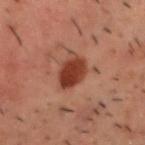<record>
  <lesion_size>
    <long_diameter_mm_approx>3.0</long_diameter_mm_approx>
  </lesion_size>
  <site>head or neck</site>
  <automated_metrics>
    <area_mm2_approx>8.0</area_mm2_approx>
    <eccentricity>0.35</eccentricity>
    <border_irregularity_0_10>1.5</border_irregularity_0_10>
    <peripheral_color_asymmetry>1.0</peripheral_color_asymmetry>
  </automated_metrics>
  <image>
    <source>total-body photography crop</source>
    <field_of_view_mm>15</field_of_view_mm>
  </image>
  <patient>
    <sex>male</sex>
    <age_approx>50</age_approx>
  </patient>
</record>